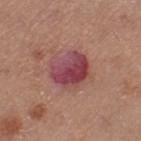<case>
<biopsy_status>not biopsied; imaged during a skin examination</biopsy_status>
<site>left thigh</site>
<patient>
  <sex>female</sex>
  <age_approx>40</age_approx>
</patient>
<lighting>white-light</lighting>
<image>
  <source>total-body photography crop</source>
  <field_of_view_mm>15</field_of_view_mm>
</image>
<lesion_size>
  <long_diameter_mm_approx>4.5</long_diameter_mm_approx>
</lesion_size>
</case>